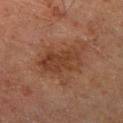Q: Is there a histopathology result?
A: no biopsy performed (imaged during a skin exam)
Q: What are the patient's age and sex?
A: male, aged approximately 60
Q: What is the lesion's diameter?
A: ~5.5 mm (longest diameter)
Q: Where on the body is the lesion?
A: the right leg
Q: What is the imaging modality?
A: ~15 mm crop, total-body skin-cancer survey